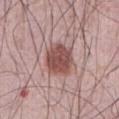Part of a total-body skin-imaging series; this lesion was reviewed on a skin check and was not flagged for biopsy. Imaged with white-light lighting. Longest diameter approximately 4 mm. A male subject, aged approximately 65. Cropped from a whole-body photographic skin survey; the tile spans about 15 mm. The lesion-visualizer software estimated an average lesion color of about L≈50 a*≈22 b*≈22 (CIELAB) and a normalized lesion–skin contrast near 9.5. It also reported peripheral color asymmetry of about 1. The lesion is located on the abdomen.